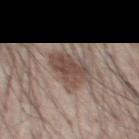Q: Was a biopsy performed?
A: no biopsy performed (imaged during a skin exam)
Q: Automated lesion metrics?
A: a mean CIELAB color near L≈48 a*≈15 b*≈23 and a normalized lesion–skin contrast near 7.5; a border-irregularity rating of about 3/10 and internal color variation of about 3.5 on a 0–10 scale; a classifier nevus-likeness of about 80/100
Q: What kind of image is this?
A: 15 mm crop, total-body photography
Q: What is the lesion's diameter?
A: ~4.5 mm (longest diameter)
Q: Patient demographics?
A: male, aged 53–57
Q: What is the anatomic site?
A: the front of the torso